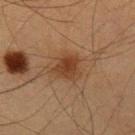biopsy_status: not biopsied; imaged during a skin examination
site: arm
image:
  source: total-body photography crop
  field_of_view_mm: 15
patient:
  sex: male
  age_approx: 35